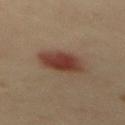biopsy_status: not biopsied; imaged during a skin examination
automated_metrics:
  area_mm2_approx: 12.0
  eccentricity: 0.75
  vs_skin_darker_L: 11.0
  vs_skin_contrast_norm: 10.0
  color_variation_0_10: 3.5
  peripheral_color_asymmetry: 1.0
  nevus_likeness_0_100: 100
  lesion_detection_confidence_0_100: 100
patient:
  sex: male
  age_approx: 40
image:
  source: total-body photography crop
  field_of_view_mm: 15
lighting: cross-polarized
site: mid back
lesion_size:
  long_diameter_mm_approx: 5.0